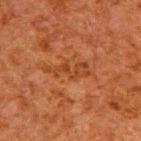Part of a total-body skin-imaging series; this lesion was reviewed on a skin check and was not flagged for biopsy.